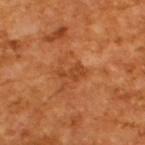This lesion was catalogued during total-body skin photography and was not selected for biopsy.
The subject is a male aged 63 to 67.
This is a cross-polarized tile.
This image is a 15 mm lesion crop taken from a total-body photograph.
Longest diameter approximately 3 mm.
An algorithmic analysis of the crop reported a footprint of about 3.5 mm², an outline eccentricity of about 0.85 (0 = round, 1 = elongated), and a shape-asymmetry score of about 0.5 (0 = symmetric). The software also gave a lesion color around L≈44 a*≈28 b*≈40 in CIELAB and a normalized lesion–skin contrast near 6. The analysis additionally found a border-irregularity rating of about 5.5/10 and a color-variation rating of about 1/10. It also reported an automated nevus-likeness rating near 0 out of 100 and a lesion-detection confidence of about 100/100.